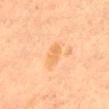Q: Is there a histopathology result?
A: imaged on a skin check; not biopsied
Q: What is the lesion's diameter?
A: ~3 mm (longest diameter)
Q: Where on the body is the lesion?
A: the abdomen
Q: What is the imaging modality?
A: ~15 mm tile from a whole-body skin photo
Q: Patient demographics?
A: female, aged approximately 60